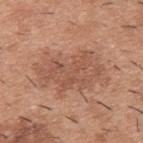Captured during whole-body skin photography for melanoma surveillance; the lesion was not biopsied.
About 7.5 mm across.
The total-body-photography lesion software estimated a mean CIELAB color near L≈54 a*≈22 b*≈30 and about 8 CIELAB-L* units darker than the surrounding skin. The analysis additionally found a border-irregularity rating of about 4.5/10 and a color-variation rating of about 3/10.
The lesion is on the back.
A roughly 15 mm field-of-view crop from a total-body skin photograph.
A male subject in their 40s.
This is a white-light tile.